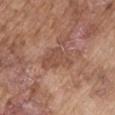body site: the arm
subject: male, in their mid- to late 70s
image source: 15 mm crop, total-body photography
size: about 4.5 mm
image-analysis metrics: a footprint of about 8.5 mm², a shape eccentricity near 0.75, and a symmetry-axis asymmetry near 0.45; a lesion–skin lightness drop of about 7; border irregularity of about 5 on a 0–10 scale, internal color variation of about 1.5 on a 0–10 scale, and peripheral color asymmetry of about 0.5
tile lighting: white-light